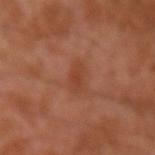Q: Was a biopsy performed?
A: total-body-photography surveillance lesion; no biopsy
Q: What are the patient's age and sex?
A: male, about 30 years old
Q: How large is the lesion?
A: ≈2.5 mm
Q: Automated lesion metrics?
A: a mean CIELAB color near L≈41 a*≈25 b*≈32, about 6 CIELAB-L* units darker than the surrounding skin, and a normalized lesion–skin contrast near 5.5; a detector confidence of about 100 out of 100 that the crop contains a lesion
Q: What lighting was used for the tile?
A: cross-polarized illumination
Q: What is the anatomic site?
A: the arm
Q: What is the imaging modality?
A: 15 mm crop, total-body photography